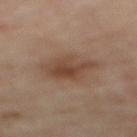Assessment: This lesion was catalogued during total-body skin photography and was not selected for biopsy. Background: The lesion-visualizer software estimated a lesion–skin lightness drop of about 8 and a lesion-to-skin contrast of about 7 (normalized; higher = more distinct). This image is a 15 mm lesion crop taken from a total-body photograph. The recorded lesion diameter is about 5 mm. The lesion is on the back. A female patient in their 60s. Imaged with cross-polarized lighting.